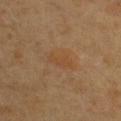site: chest
lighting: cross-polarized
patient:
  sex: female
  age_approx: 40
image:
  source: total-body photography crop
  field_of_view_mm: 15
lesion_size:
  long_diameter_mm_approx: 3.5